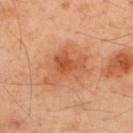No biopsy was performed on this lesion — it was imaged during a full skin examination and was not determined to be concerning. The lesion is located on the back. A roughly 15 mm field-of-view crop from a total-body skin photograph. A male subject, aged 48–52. Longest diameter approximately 6 mm. Imaged with cross-polarized lighting. Automated image analysis of the tile measured a footprint of about 18 mm², an outline eccentricity of about 0.65 (0 = round, 1 = elongated), and a shape-asymmetry score of about 0.3 (0 = symmetric). It also reported a border-irregularity rating of about 3.5/10 and a within-lesion color-variation index near 5.5/10.